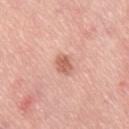  biopsy_status: not biopsied; imaged during a skin examination
  image:
    source: total-body photography crop
    field_of_view_mm: 15
  patient:
    sex: male
    age_approx: 70
  site: leg